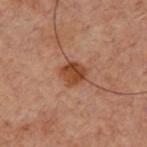  biopsy_status: not biopsied; imaged during a skin examination
  lighting: cross-polarized
  image:
    source: total-body photography crop
    field_of_view_mm: 15
  lesion_size:
    long_diameter_mm_approx: 3.5
  patient:
    sex: male
    age_approx: 60
  site: chest